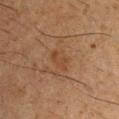{
  "biopsy_status": "not biopsied; imaged during a skin examination",
  "patient": {
    "sex": "male",
    "age_approx": 35
  },
  "automated_metrics": {
    "cielab_L": 33,
    "cielab_a": 17,
    "cielab_b": 27,
    "vs_skin_darker_L": 5.0,
    "vs_skin_contrast_norm": 5.5
  },
  "site": "left upper arm",
  "image": {
    "source": "total-body photography crop",
    "field_of_view_mm": 15
  },
  "lesion_size": {
    "long_diameter_mm_approx": 3.0
  }
}